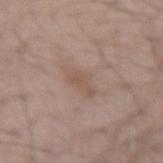| key | value |
|---|---|
| biopsy status | catalogued during a skin exam; not biopsied |
| site | the left forearm |
| patient | male, aged around 55 |
| acquisition | ~15 mm crop, total-body skin-cancer survey |